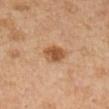Clinical impression:
Captured during whole-body skin photography for melanoma surveillance; the lesion was not biopsied.
Image and clinical context:
Measured at roughly 2.5 mm in maximum diameter. The patient is a female approximately 40 years of age. Located on the left forearm. A 15 mm crop from a total-body photograph taken for skin-cancer surveillance.